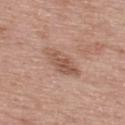Findings:
* follow-up · no biopsy performed (imaged during a skin exam)
* image · 15 mm crop, total-body photography
* image-analysis metrics · a mean CIELAB color near L≈55 a*≈20 b*≈28 and a normalized border contrast of about 6.5
* lesion size · about 5 mm
* subject · male, aged around 65
* site · the upper back